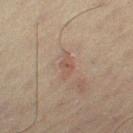The lesion is on the right thigh.
A male subject roughly 65 years of age.
Cropped from a total-body skin-imaging series; the visible field is about 15 mm.